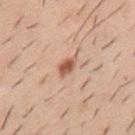The lesion was tiled from a total-body skin photograph and was not biopsied. Imaged with white-light lighting. Automated image analysis of the tile measured an average lesion color of about L≈58 a*≈22 b*≈32 (CIELAB). The analysis additionally found a nevus-likeness score of about 90/100. A male subject aged around 40. Longest diameter approximately 3 mm. The lesion is on the mid back. A close-up tile cropped from a whole-body skin photograph, about 15 mm across.